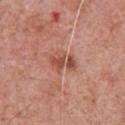Impression: Part of a total-body skin-imaging series; this lesion was reviewed on a skin check and was not flagged for biopsy. Image and clinical context: The lesion is on the chest. The patient is a male about 70 years old. A 15 mm close-up tile from a total-body photography series done for melanoma screening.